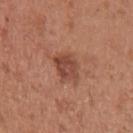Imaged during a routine full-body skin examination; the lesion was not biopsied and no histopathology is available.
On the left upper arm.
A 15 mm close-up extracted from a 3D total-body photography capture.
A female subject, aged 28–32.